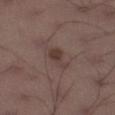Clinical impression: Imaged during a routine full-body skin examination; the lesion was not biopsied and no histopathology is available. Context: The patient is a male about 40 years old. Located on the left lower leg. Longest diameter approximately 3 mm. A close-up tile cropped from a whole-body skin photograph, about 15 mm across.